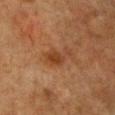Captured during whole-body skin photography for melanoma surveillance; the lesion was not biopsied. The total-body-photography lesion software estimated a lesion area of about 6 mm², an eccentricity of roughly 0.85, and two-axis asymmetry of about 0.4. The software also gave an average lesion color of about L≈34 a*≈20 b*≈30 (CIELAB) and a normalized border contrast of about 7. The analysis additionally found a border-irregularity rating of about 4.5/10, internal color variation of about 2.5 on a 0–10 scale, and a peripheral color-asymmetry measure near 1. About 4 mm across. This is a cross-polarized tile. Cropped from a total-body skin-imaging series; the visible field is about 15 mm. A female patient aged 53–57. Located on the head or neck.